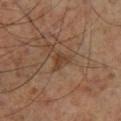Part of a total-body skin-imaging series; this lesion was reviewed on a skin check and was not flagged for biopsy.
Imaged with cross-polarized lighting.
The lesion is located on the right lower leg.
Automated image analysis of the tile measured an eccentricity of roughly 0.55. The software also gave border irregularity of about 5 on a 0–10 scale, internal color variation of about 1.5 on a 0–10 scale, and peripheral color asymmetry of about 0.5.
Approximately 2.5 mm at its widest.
A roughly 15 mm field-of-view crop from a total-body skin photograph.
A male patient, about 60 years old.